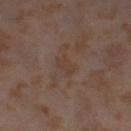biopsy status: imaged on a skin check; not biopsied
site: the right thigh
size: ~2.5 mm (longest diameter)
subject: female, in their mid-50s
automated lesion analysis: an outline eccentricity of about 0.7 (0 = round, 1 = elongated) and a symmetry-axis asymmetry near 0.3; a within-lesion color-variation index near 1/10 and radial color variation of about 0.5; a lesion-detection confidence of about 100/100
tile lighting: cross-polarized
image source: total-body-photography crop, ~15 mm field of view